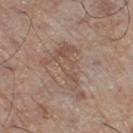<record>
<biopsy_status>not biopsied; imaged during a skin examination</biopsy_status>
<lesion_size>
  <long_diameter_mm_approx>6.5</long_diameter_mm_approx>
</lesion_size>
<site>left lower leg</site>
<automated_metrics>
  <cielab_L>52</cielab_L>
  <cielab_a>16</cielab_a>
  <cielab_b>26</cielab_b>
  <vs_skin_darker_L>7.0</vs_skin_darker_L>
  <vs_skin_contrast_norm>5.5</vs_skin_contrast_norm>
  <nevus_likeness_0_100>0</nevus_likeness_0_100>
  <lesion_detection_confidence_0_100>95</lesion_detection_confidence_0_100>
</automated_metrics>
<lighting>white-light</lighting>
<patient>
  <sex>male</sex>
  <age_approx>70</age_approx>
</patient>
<image>
  <source>total-body photography crop</source>
  <field_of_view_mm>15</field_of_view_mm>
</image>
</record>